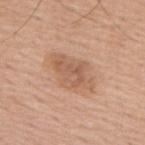| field | value |
|---|---|
| workup | imaged on a skin check; not biopsied |
| lesion diameter | ~5.5 mm (longest diameter) |
| subject | male, aged 73–77 |
| tile lighting | white-light illumination |
| imaging modality | total-body-photography crop, ~15 mm field of view |
| automated lesion analysis | an area of roughly 14 mm², a shape eccentricity near 0.8, and a symmetry-axis asymmetry near 0.2; border irregularity of about 3 on a 0–10 scale and a within-lesion color-variation index near 4/10; an automated nevus-likeness rating near 20 out of 100 and a lesion-detection confidence of about 100/100 |
| site | the upper back |